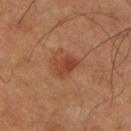biopsy_status: not biopsied; imaged during a skin examination
lighting: cross-polarized
patient:
  sex: male
  age_approx: 50
image:
  source: total-body photography crop
  field_of_view_mm: 15
lesion_size:
  long_diameter_mm_approx: 3.0
automated_metrics:
  area_mm2_approx: 5.0
  eccentricity: 0.75
  shape_asymmetry: 0.25
  border_irregularity_0_10: 2.5
  color_variation_0_10: 3.5
  nevus_likeness_0_100: 80
  lesion_detection_confidence_0_100: 100
site: right upper arm